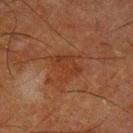Clinical summary:
Automated tile analysis of the lesion measured a footprint of about 7 mm², an outline eccentricity of about 0.7 (0 = round, 1 = elongated), and a shape-asymmetry score of about 0.35 (0 = symmetric). The analysis additionally found a lesion color around L≈29 a*≈20 b*≈27 in CIELAB, about 5 CIELAB-L* units darker than the surrounding skin, and a normalized border contrast of about 5.5. And it measured a detector confidence of about 100 out of 100 that the crop contains a lesion. Longest diameter approximately 3.5 mm. A male subject, approximately 65 years of age. Captured under cross-polarized illumination. Cropped from a whole-body photographic skin survey; the tile spans about 15 mm. On the left lower leg.